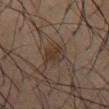Assessment: This lesion was catalogued during total-body skin photography and was not selected for biopsy. Image and clinical context: A male patient in their mid- to late 50s. A 15 mm close-up tile from a total-body photography series done for melanoma screening. About 2.5 mm across. The lesion is located on the abdomen. Automated tile analysis of the lesion measured a color-variation rating of about 4.5/10.